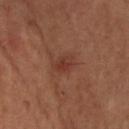Q: Is there a histopathology result?
A: total-body-photography surveillance lesion; no biopsy
Q: What is the anatomic site?
A: the upper back
Q: What is the lesion's diameter?
A: ~2.5 mm (longest diameter)
Q: Illumination type?
A: cross-polarized illumination
Q: What kind of image is this?
A: ~15 mm crop, total-body skin-cancer survey
Q: Who is the patient?
A: male, aged 33–37